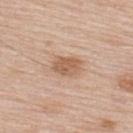| key | value |
|---|---|
| follow-up | total-body-photography surveillance lesion; no biopsy |
| patient | male, aged around 80 |
| imaging modality | total-body-photography crop, ~15 mm field of view |
| lesion diameter | about 3 mm |
| illumination | white-light |
| body site | the back |
| image-analysis metrics | a border-irregularity rating of about 2/10 and a within-lesion color-variation index near 2/10; an automated nevus-likeness rating near 50 out of 100 and a lesion-detection confidence of about 100/100 |